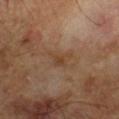The lesion was photographed on a routine skin check and not biopsied; there is no pathology result.
Captured under cross-polarized illumination.
A male subject aged 63–67.
A region of skin cropped from a whole-body photographic capture, roughly 15 mm wide.
Measured at roughly 2.5 mm in maximum diameter.